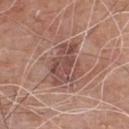biopsy status: no biopsy performed (imaged during a skin exam); acquisition: 15 mm crop, total-body photography; anatomic site: the chest; patient: male, approximately 80 years of age.